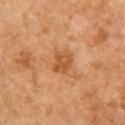biopsy status: total-body-photography surveillance lesion; no biopsy
imaging modality: total-body-photography crop, ~15 mm field of view
patient: female, aged 58 to 62
anatomic site: the right upper arm